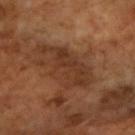* biopsy status · no biopsy performed (imaged during a skin exam)
* site · the right forearm
* patient · female, aged 68–72
* acquisition · total-body-photography crop, ~15 mm field of view